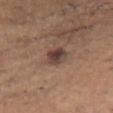  biopsy_status: not biopsied; imaged during a skin examination
  patient:
    sex: female
    age_approx: 40
  lesion_size:
    long_diameter_mm_approx: 2.5
  lighting: white-light
  image:
    source: total-body photography crop
    field_of_view_mm: 15
  site: chest